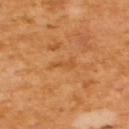The lesion was photographed on a routine skin check and not biopsied; there is no pathology result.
An algorithmic analysis of the crop reported a footprint of about 2 mm², an eccentricity of roughly 0.95, and a symmetry-axis asymmetry near 0.4. It also reported an average lesion color of about L≈51 a*≈26 b*≈43 (CIELAB), roughly 7 lightness units darker than nearby skin, and a normalized lesion–skin contrast near 5.
Imaged with cross-polarized lighting.
Approximately 2.5 mm at its widest.
A male subject, aged around 65.
A lesion tile, about 15 mm wide, cut from a 3D total-body photograph.